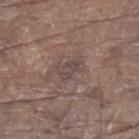{"biopsy_status": "not biopsied; imaged during a skin examination", "automated_metrics": {"cielab_L": 44, "cielab_a": 14, "cielab_b": 19, "vs_skin_darker_L": 6.0, "vs_skin_contrast_norm": 5.5, "nevus_likeness_0_100": 0, "lesion_detection_confidence_0_100": 65}, "site": "left lower leg", "patient": {"sex": "male", "age_approx": 80}, "lighting": "white-light", "image": {"source": "total-body photography crop", "field_of_view_mm": 15}}